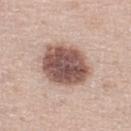biopsy status: total-body-photography surveillance lesion; no biopsy
lesion size: ≈6 mm
TBP lesion metrics: a border-irregularity index near 1.5/10 and peripheral color asymmetry of about 2; an automated nevus-likeness rating near 20 out of 100 and lesion-presence confidence of about 100/100
location: the left lower leg
subject: male, aged approximately 65
tile lighting: white-light illumination
image: 15 mm crop, total-body photography